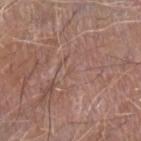Imaged during a routine full-body skin examination; the lesion was not biopsied and no histopathology is available.
A close-up tile cropped from a whole-body skin photograph, about 15 mm across.
The subject is a male aged approximately 65.
The tile uses white-light illumination.
About 1 mm across.
The lesion is on the left forearm.